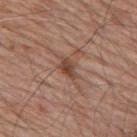{"biopsy_status": "not biopsied; imaged during a skin examination", "lesion_size": {"long_diameter_mm_approx": 3.0}, "automated_metrics": {"nevus_likeness_0_100": 10, "lesion_detection_confidence_0_100": 100}, "patient": {"sex": "male", "age_approx": 80}, "image": {"source": "total-body photography crop", "field_of_view_mm": 15}, "site": "mid back", "lighting": "white-light"}